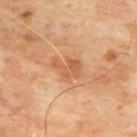A male subject aged 63–67. A roughly 15 mm field-of-view crop from a total-body skin photograph. The lesion is on the upper back.